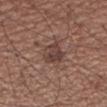<record>
<biopsy_status>not biopsied; imaged during a skin examination</biopsy_status>
<image>
  <source>total-body photography crop</source>
  <field_of_view_mm>15</field_of_view_mm>
</image>
<patient>
  <sex>male</sex>
  <age_approx>50</age_approx>
</patient>
<lesion_size>
  <long_diameter_mm_approx>3.5</long_diameter_mm_approx>
</lesion_size>
<site>right upper arm</site>
<lighting>white-light</lighting>
</record>